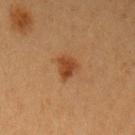Imaged during a routine full-body skin examination; the lesion was not biopsied and no histopathology is available.